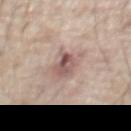Impression: The lesion was tiled from a total-body skin photograph and was not biopsied. Clinical summary: The lesion is on the chest. A lesion tile, about 15 mm wide, cut from a 3D total-body photograph. The tile uses white-light illumination. The subject is a male aged approximately 80.